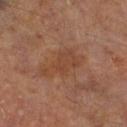Impression:
Imaged during a routine full-body skin examination; the lesion was not biopsied and no histopathology is available.
Clinical summary:
An algorithmic analysis of the crop reported a lesion area of about 9 mm², an outline eccentricity of about 0.85 (0 = round, 1 = elongated), and a symmetry-axis asymmetry near 0.55. The analysis additionally found a border-irregularity rating of about 6.5/10, a within-lesion color-variation index near 1.5/10, and peripheral color asymmetry of about 0.5. The subject is a male aged 68–72. This is a cross-polarized tile. The recorded lesion diameter is about 5 mm. This image is a 15 mm lesion crop taken from a total-body photograph. The lesion is on the right lower leg.